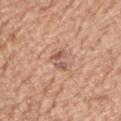Part of a total-body skin-imaging series; this lesion was reviewed on a skin check and was not flagged for biopsy.
A 15 mm close-up extracted from a 3D total-body photography capture.
The subject is a female aged 73–77.
The lesion is located on the left upper arm.
Captured under white-light illumination.
The lesion-visualizer software estimated a lesion color around L≈56 a*≈23 b*≈28 in CIELAB and about 10 CIELAB-L* units darker than the surrounding skin. The software also gave a classifier nevus-likeness of about 5/100 and a detector confidence of about 100 out of 100 that the crop contains a lesion.